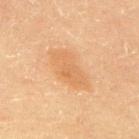follow-up — no biopsy performed (imaged during a skin exam)
size — ~5.5 mm (longest diameter)
illumination — cross-polarized
subject — female, in their mid-60s
image-analysis metrics — a lesion color around L≈57 a*≈19 b*≈37 in CIELAB, a lesion–skin lightness drop of about 6, and a normalized border contrast of about 5; a classifier nevus-likeness of about 35/100 and a lesion-detection confidence of about 100/100
anatomic site — the upper back
acquisition — ~15 mm tile from a whole-body skin photo